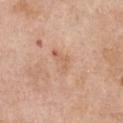Clinical impression: The lesion was tiled from a total-body skin photograph and was not biopsied.